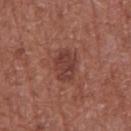Image and clinical context:
A male subject, in their mid-60s. On the abdomen. This is a white-light tile. About 3.5 mm across. Automated image analysis of the tile measured an eccentricity of roughly 0.7 and a shape-asymmetry score of about 0.15 (0 = symmetric). The analysis additionally found an average lesion color of about L≈40 a*≈24 b*≈24 (CIELAB) and a normalized lesion–skin contrast near 7. A roughly 15 mm field-of-view crop from a total-body skin photograph.